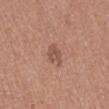notes=catalogued during a skin exam; not biopsied
diameter=≈2.5 mm
image=~15 mm crop, total-body skin-cancer survey
patient=female, roughly 50 years of age
body site=the right lower leg